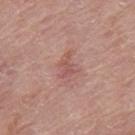Findings:
* notes — catalogued during a skin exam; not biopsied
* lesion size — ≈3 mm
* acquisition — total-body-photography crop, ~15 mm field of view
* subject — female, about 60 years old
* body site — the right thigh
* illumination — white-light illumination